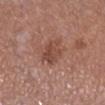Assessment:
The lesion was tiled from a total-body skin photograph and was not biopsied.
Clinical summary:
A roughly 15 mm field-of-view crop from a total-body skin photograph. The tile uses white-light illumination. The subject is a female approximately 60 years of age. The lesion is on the right lower leg.